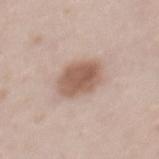Captured during whole-body skin photography for melanoma surveillance; the lesion was not biopsied.